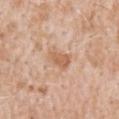Impression:
The lesion was tiled from a total-body skin photograph and was not biopsied.
Clinical summary:
Automated tile analysis of the lesion measured an area of roughly 5.5 mm², an eccentricity of roughly 0.8, and two-axis asymmetry of about 0.25. It also reported a lesion color around L≈61 a*≈21 b*≈33 in CIELAB, a lesion–skin lightness drop of about 9, and a lesion-to-skin contrast of about 6.5 (normalized; higher = more distinct). About 3 mm across. Imaged with white-light lighting. The patient is a male aged 48–52. A region of skin cropped from a whole-body photographic capture, roughly 15 mm wide. On the mid back.